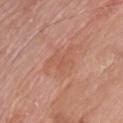Findings:
* biopsy status · catalogued during a skin exam; not biopsied
* lesion size · ~4.5 mm (longest diameter)
* location · the chest
* subject · male, aged 58–62
* tile lighting · white-light
* TBP lesion metrics · an area of roughly 10 mm², an outline eccentricity of about 0.6 (0 = round, 1 = elongated), and a shape-asymmetry score of about 0.45 (0 = symmetric); an average lesion color of about L≈57 a*≈24 b*≈31 (CIELAB) and about 6 CIELAB-L* units darker than the surrounding skin
* image · 15 mm crop, total-body photography